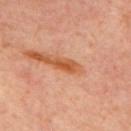Q: Is there a histopathology result?
A: total-body-photography surveillance lesion; no biopsy
Q: What kind of image is this?
A: ~15 mm crop, total-body skin-cancer survey
Q: How large is the lesion?
A: ~2.5 mm (longest diameter)
Q: Who is the patient?
A: male, about 65 years old
Q: Where on the body is the lesion?
A: the back
Q: What lighting was used for the tile?
A: cross-polarized